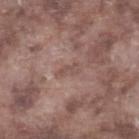Case summary:
• notes · total-body-photography surveillance lesion; no biopsy
• patient · male, roughly 75 years of age
• image-analysis metrics · a lesion color around L≈50 a*≈18 b*≈22 in CIELAB, a lesion–skin lightness drop of about 6, and a normalized border contrast of about 4.5; an automated nevus-likeness rating near 0 out of 100 and a lesion-detection confidence of about 80/100
• acquisition · ~15 mm crop, total-body skin-cancer survey
• body site · the right lower leg
• lesion size · ~2.5 mm (longest diameter)
• lighting · white-light